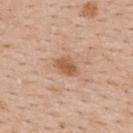biopsy status — total-body-photography surveillance lesion; no biopsy
site — the back
acquisition — ~15 mm tile from a whole-body skin photo
subject — female, in their mid- to late 40s